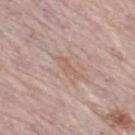Part of a total-body skin-imaging series; this lesion was reviewed on a skin check and was not flagged for biopsy.
The lesion's longest dimension is about 3.5 mm.
Imaged with white-light lighting.
On the left leg.
A region of skin cropped from a whole-body photographic capture, roughly 15 mm wide.
The patient is a female roughly 65 years of age.